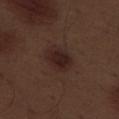Recorded during total-body skin imaging; not selected for excision or biopsy. On the left thigh. A male patient, in their 70s. Cropped from a whole-body photographic skin survey; the tile spans about 15 mm.